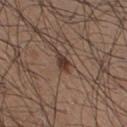No biopsy was performed on this lesion — it was imaged during a full skin examination and was not determined to be concerning.
Captured under white-light illumination.
A male patient aged around 35.
A roughly 15 mm field-of-view crop from a total-body skin photograph.
The lesion is on the right thigh.
An algorithmic analysis of the crop reported a border-irregularity rating of about 3/10, a color-variation rating of about 3/10, and peripheral color asymmetry of about 1.